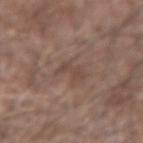This lesion was catalogued during total-body skin photography and was not selected for biopsy.
An algorithmic analysis of the crop reported a border-irregularity rating of about 5/10 and internal color variation of about 0.5 on a 0–10 scale.
The patient is a male aged 58 to 62.
From the left forearm.
The recorded lesion diameter is about 2.5 mm.
A 15 mm close-up extracted from a 3D total-body photography capture.